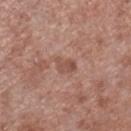biopsy_status: not biopsied; imaged during a skin examination
patient:
  sex: male
  age_approx: 55
site: right lower leg
image:
  source: total-body photography crop
  field_of_view_mm: 15
lighting: white-light